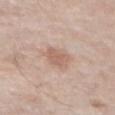workup = catalogued during a skin exam; not biopsied
subject = male, aged approximately 80
imaging modality = ~15 mm crop, total-body skin-cancer survey
site = the chest
diameter = ~3.5 mm (longest diameter)
automated metrics = an eccentricity of roughly 0.75 and a symmetry-axis asymmetry near 0.3; a mean CIELAB color near L≈61 a*≈19 b*≈27 and roughly 9 lightness units darker than nearby skin; an automated nevus-likeness rating near 5 out of 100 and lesion-presence confidence of about 100/100